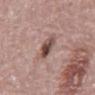No biopsy was performed on this lesion — it was imaged during a full skin examination and was not determined to be concerning. The subject is a male about 70 years old. The lesion is on the back. A close-up tile cropped from a whole-body skin photograph, about 15 mm across.